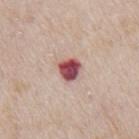{"biopsy_status": "not biopsied; imaged during a skin examination", "lighting": "white-light", "site": "mid back", "automated_metrics": {"area_mm2_approx": 5.5, "eccentricity": 0.35, "border_irregularity_0_10": 2.0}, "patient": {"sex": "male", "age_approx": 65}, "image": {"source": "total-body photography crop", "field_of_view_mm": 15}}